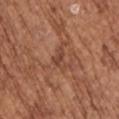The subject is a female in their mid-70s. Located on the left upper arm. A region of skin cropped from a whole-body photographic capture, roughly 15 mm wide.